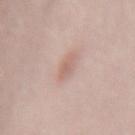{"biopsy_status": "not biopsied; imaged during a skin examination", "lighting": "white-light", "image": {"source": "total-body photography crop", "field_of_view_mm": 15}, "lesion_size": {"long_diameter_mm_approx": 2.5}, "patient": {"sex": "female", "age_approx": 50}, "site": "mid back"}